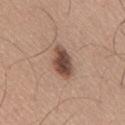Assessment: Part of a total-body skin-imaging series; this lesion was reviewed on a skin check and was not flagged for biopsy. Context: Cropped from a whole-body photographic skin survey; the tile spans about 15 mm. On the lower back. A male patient in their mid-60s.